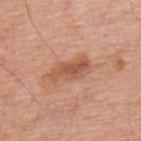  patient:
    sex: male
    age_approx: 60
  image:
    source: total-body photography crop
    field_of_view_mm: 15
  automated_metrics:
    border_irregularity_0_10: 4.0
    color_variation_0_10: 3.5
    peripheral_color_asymmetry: 1.0
  lighting: white-light
  site: upper back
  lesion_size:
    long_diameter_mm_approx: 5.0